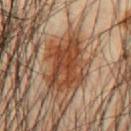The lesion was photographed on a routine skin check and not biopsied; there is no pathology result.
Approximately 7 mm at its widest.
The lesion-visualizer software estimated a lesion area of about 23 mm² and a symmetry-axis asymmetry near 0.25. It also reported a mean CIELAB color near L≈43 a*≈22 b*≈32 and about 11 CIELAB-L* units darker than the surrounding skin.
The subject is a male roughly 45 years of age.
On the chest.
A roughly 15 mm field-of-view crop from a total-body skin photograph.